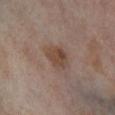Captured during whole-body skin photography for melanoma surveillance; the lesion was not biopsied.
The lesion's longest dimension is about 3 mm.
Captured under cross-polarized illumination.
Cropped from a whole-body photographic skin survey; the tile spans about 15 mm.
A female patient, aged around 55.
From the leg.